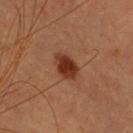{
  "biopsy_status": "not biopsied; imaged during a skin examination",
  "site": "upper back",
  "lesion_size": {
    "long_diameter_mm_approx": 3.5
  },
  "patient": {
    "sex": "female",
    "age_approx": 40
  },
  "image": {
    "source": "total-body photography crop",
    "field_of_view_mm": 15
  }
}